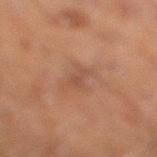Clinical summary: A region of skin cropped from a whole-body photographic capture, roughly 15 mm wide. The total-body-photography lesion software estimated about 5 CIELAB-L* units darker than the surrounding skin and a normalized lesion–skin contrast near 5. This is a cross-polarized tile. A male patient about 50 years old. Measured at roughly 3 mm in maximum diameter. The lesion is located on the right lower leg.